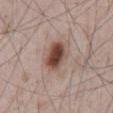notes: imaged on a skin check; not biopsied
TBP lesion metrics: a shape-asymmetry score of about 0.2 (0 = symmetric); an average lesion color of about L≈47 a*≈19 b*≈25 (CIELAB) and a lesion-to-skin contrast of about 11 (normalized; higher = more distinct); a within-lesion color-variation index near 7.5/10; a nevus-likeness score of about 100/100
image: total-body-photography crop, ~15 mm field of view
lesion size: ~4.5 mm (longest diameter)
tile lighting: white-light illumination
patient: male, about 65 years old
body site: the abdomen